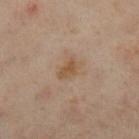Clinical summary:
A female subject in their 60s. Longest diameter approximately 3 mm. A close-up tile cropped from a whole-body skin photograph, about 15 mm across. The lesion is located on the right leg. This is a cross-polarized tile.